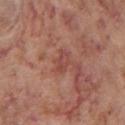Q: Who is the patient?
A: male, about 55 years old
Q: Illumination type?
A: white-light illumination
Q: What is the anatomic site?
A: the chest
Q: How was this image acquired?
A: ~15 mm crop, total-body skin-cancer survey
Q: Lesion size?
A: ≈2.5 mm